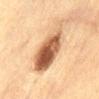Impression: The lesion was tiled from a total-body skin photograph and was not biopsied. Clinical summary: Imaged with cross-polarized lighting. Cropped from a whole-body photographic skin survey; the tile spans about 15 mm. The lesion-visualizer software estimated an eccentricity of roughly 0.8 and a symmetry-axis asymmetry near 0.25. It also reported a classifier nevus-likeness of about 90/100 and a detector confidence of about 100 out of 100 that the crop contains a lesion. The subject is a male aged 58 to 62. The lesion is located on the abdomen.